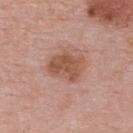Notes:
- notes — no biopsy performed (imaged during a skin exam)
- automated lesion analysis — a lesion area of about 12 mm², an outline eccentricity of about 0.7 (0 = round, 1 = elongated), and a shape-asymmetry score of about 0.25 (0 = symmetric); an average lesion color of about L≈53 a*≈22 b*≈30 (CIELAB), roughly 10 lightness units darker than nearby skin, and a normalized lesion–skin contrast near 8; a border-irregularity rating of about 2.5/10 and internal color variation of about 3.5 on a 0–10 scale
- lesion diameter — ≈4.5 mm
- location — the upper back
- image — total-body-photography crop, ~15 mm field of view
- patient — female, about 50 years old